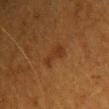The lesion was photographed on a routine skin check and not biopsied; there is no pathology result.
A 15 mm close-up tile from a total-body photography series done for melanoma screening.
Located on the front of the torso.
The tile uses cross-polarized illumination.
The subject is a female aged 38 to 42.
The total-body-photography lesion software estimated a lesion area of about 5.5 mm², a shape eccentricity near 0.8, and two-axis asymmetry of about 0.3.